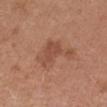• workup — no biopsy performed (imaged during a skin exam)
• automated metrics — a mean CIELAB color near L≈50 a*≈23 b*≈30 and about 8 CIELAB-L* units darker than the surrounding skin
• image source — total-body-photography crop, ~15 mm field of view
• illumination — white-light
• patient — female, about 45 years old
• location — the arm
• lesion size — about 6 mm